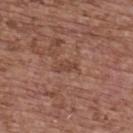biopsy_status: not biopsied; imaged during a skin examination
lesion_size:
  long_diameter_mm_approx: 2.5
image:
  source: total-body photography crop
  field_of_view_mm: 15
lighting: white-light
site: upper back
patient:
  sex: female
  age_approx: 65